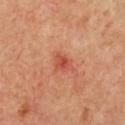| key | value |
|---|---|
| follow-up | catalogued during a skin exam; not biopsied |
| site | the chest |
| patient | female, aged around 40 |
| imaging modality | total-body-photography crop, ~15 mm field of view |